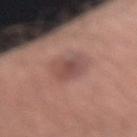The lesion was tiled from a total-body skin photograph and was not biopsied.
A male patient aged around 45.
Captured under white-light illumination.
The total-body-photography lesion software estimated a lesion color around L≈49 a*≈21 b*≈23 in CIELAB, about 9 CIELAB-L* units darker than the surrounding skin, and a normalized lesion–skin contrast near 6.5. The analysis additionally found an automated nevus-likeness rating near 45 out of 100 and lesion-presence confidence of about 100/100.
This image is a 15 mm lesion crop taken from a total-body photograph.
Approximately 4 mm at its widest.
From the right forearm.